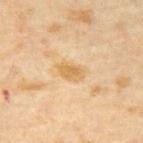Clinical impression:
Part of a total-body skin-imaging series; this lesion was reviewed on a skin check and was not flagged for biopsy.
Background:
A male patient, about 65 years old. A 15 mm close-up extracted from a 3D total-body photography capture.